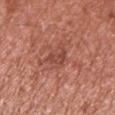Assessment:
Captured during whole-body skin photography for melanoma surveillance; the lesion was not biopsied.
Acquisition and patient details:
A 15 mm close-up extracted from a 3D total-body photography capture. The subject is a male in their mid-60s. Captured under white-light illumination. The lesion is on the left upper arm. The total-body-photography lesion software estimated a footprint of about 3 mm², an eccentricity of roughly 0.75, and a symmetry-axis asymmetry near 0.4. The analysis additionally found a mean CIELAB color near L≈46 a*≈27 b*≈28, about 8 CIELAB-L* units darker than the surrounding skin, and a lesion-to-skin contrast of about 6 (normalized; higher = more distinct). And it measured border irregularity of about 4 on a 0–10 scale, a color-variation rating of about 1.5/10, and a peripheral color-asymmetry measure near 0.5. The recorded lesion diameter is about 2.5 mm.